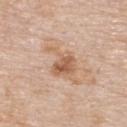Impression: Part of a total-body skin-imaging series; this lesion was reviewed on a skin check and was not flagged for biopsy. Image and clinical context: The lesion is located on the upper back. The lesion-visualizer software estimated an average lesion color of about L≈60 a*≈20 b*≈33 (CIELAB) and roughly 10 lightness units darker than nearby skin. The software also gave border irregularity of about 5.5 on a 0–10 scale, internal color variation of about 4.5 on a 0–10 scale, and radial color variation of about 1.5. The lesion's longest dimension is about 4.5 mm. A male subject in their mid- to late 80s or older. Captured under white-light illumination. A roughly 15 mm field-of-view crop from a total-body skin photograph.